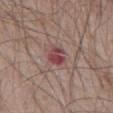<record>
  <biopsy_status>not biopsied; imaged during a skin examination</biopsy_status>
  <patient>
    <sex>male</sex>
    <age_approx>65</age_approx>
  </patient>
  <site>abdomen</site>
  <image>
    <source>total-body photography crop</source>
    <field_of_view_mm>15</field_of_view_mm>
  </image>
</record>